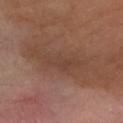{
  "biopsy_status": "not biopsied; imaged during a skin examination",
  "image": {
    "source": "total-body photography crop",
    "field_of_view_mm": 15
  },
  "patient": {
    "sex": "male",
    "age_approx": 55
  },
  "lighting": "cross-polarized",
  "lesion_size": {
    "long_diameter_mm_approx": 8.5
  },
  "automated_metrics": {
    "area_mm2_approx": 19.0,
    "eccentricity": 0.95,
    "shape_asymmetry": 0.35,
    "cielab_L": 43,
    "cielab_a": 19,
    "cielab_b": 27,
    "vs_skin_darker_L": 6.0,
    "vs_skin_contrast_norm": 5.0,
    "border_irregularity_0_10": 6.0,
    "color_variation_0_10": 2.5
  },
  "site": "right forearm"
}